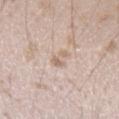This lesion was catalogued during total-body skin photography and was not selected for biopsy.
A close-up tile cropped from a whole-body skin photograph, about 15 mm across.
A male subject, aged 23–27.
The lesion is located on the left forearm.